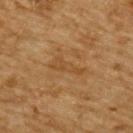Clinical impression:
No biopsy was performed on this lesion — it was imaged during a full skin examination and was not determined to be concerning.
Context:
Measured at roughly 4 mm in maximum diameter. This is a cross-polarized tile. From the back. Cropped from a total-body skin-imaging series; the visible field is about 15 mm. The subject is a male aged 83 or older.